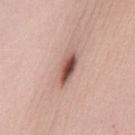Imaged during a routine full-body skin examination; the lesion was not biopsied and no histopathology is available. The patient is a female aged 23–27. The lesion is located on the chest. Measured at roughly 4.5 mm in maximum diameter. Cropped from a whole-body photographic skin survey; the tile spans about 15 mm.